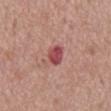Captured during whole-body skin photography for melanoma surveillance; the lesion was not biopsied. The lesion is on the back. About 3 mm across. The patient is a male aged around 65. A lesion tile, about 15 mm wide, cut from a 3D total-body photograph. This is a white-light tile.